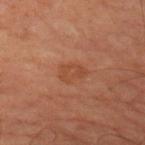{
  "biopsy_status": "not biopsied; imaged during a skin examination",
  "image": {
    "source": "total-body photography crop",
    "field_of_view_mm": 15
  },
  "site": "right upper arm",
  "patient": {
    "sex": "male",
    "age_approx": 70
  },
  "lesion_size": {
    "long_diameter_mm_approx": 3.0
  },
  "automated_metrics": {
    "area_mm2_approx": 5.5,
    "eccentricity": 0.7,
    "shape_asymmetry": 0.25,
    "cielab_L": 35,
    "cielab_a": 20,
    "cielab_b": 26,
    "vs_skin_darker_L": 4.0,
    "vs_skin_contrast_norm": 4.5,
    "border_irregularity_0_10": 2.5,
    "color_variation_0_10": 1.5,
    "peripheral_color_asymmetry": 0.5
  },
  "lighting": "cross-polarized"
}